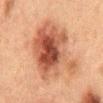follow-up — total-body-photography surveillance lesion; no biopsy